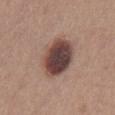| feature | finding |
|---|---|
| biopsy status | catalogued during a skin exam; not biopsied |
| anatomic site | the front of the torso |
| image source | ~15 mm crop, total-body skin-cancer survey |
| lesion diameter | ~5.5 mm (longest diameter) |
| patient | male, in their mid- to late 20s |